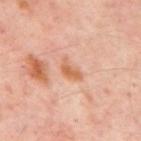Imaged with cross-polarized lighting.
About 3 mm across.
A subject approximately 55 years of age.
A 15 mm close-up extracted from a 3D total-body photography capture.
The lesion is on the mid back.